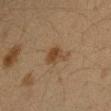Q: Was this lesion biopsied?
A: no biopsy performed (imaged during a skin exam)
Q: What lighting was used for the tile?
A: cross-polarized
Q: What is the imaging modality?
A: 15 mm crop, total-body photography
Q: Automated lesion metrics?
A: a lesion area of about 5 mm² and two-axis asymmetry of about 0.35; an average lesion color of about L≈35 a*≈14 b*≈28 (CIELAB), a lesion–skin lightness drop of about 8, and a normalized border contrast of about 7.5
Q: How large is the lesion?
A: about 3.5 mm
Q: What is the anatomic site?
A: the left forearm
Q: Who is the patient?
A: female, about 40 years old